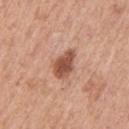Recorded during total-body skin imaging; not selected for excision or biopsy.
A male subject aged around 75.
On the left upper arm.
A 15 mm crop from a total-body photograph taken for skin-cancer surveillance.